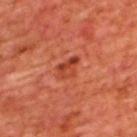Q: Was this lesion biopsied?
A: total-body-photography surveillance lesion; no biopsy
Q: What lighting was used for the tile?
A: cross-polarized
Q: Lesion size?
A: ~3 mm (longest diameter)
Q: What is the anatomic site?
A: the upper back
Q: What are the patient's age and sex?
A: male, aged around 65
Q: What is the imaging modality?
A: ~15 mm crop, total-body skin-cancer survey